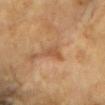This lesion was catalogued during total-body skin photography and was not selected for biopsy.
A region of skin cropped from a whole-body photographic capture, roughly 15 mm wide.
Imaged with cross-polarized lighting.
On the head or neck.
A female patient, aged approximately 60.
The total-body-photography lesion software estimated a lesion area of about 4 mm² and a shape-asymmetry score of about 0.5 (0 = symmetric). The analysis additionally found a nevus-likeness score of about 0/100 and lesion-presence confidence of about 90/100.